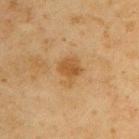The tile uses cross-polarized illumination.
The patient is a male aged approximately 45.
A roughly 15 mm field-of-view crop from a total-body skin photograph.
Approximately 3.5 mm at its widest.
The lesion is on the right upper arm.